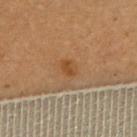– biopsy status: no biopsy performed (imaged during a skin exam)
– body site: the upper back
– subject: female, approximately 65 years of age
– image: ~15 mm tile from a whole-body skin photo
– lighting: cross-polarized illumination